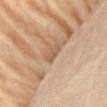Imaged during a routine full-body skin examination; the lesion was not biopsied and no histopathology is available. The lesion is on the abdomen. A 15 mm close-up tile from a total-body photography series done for melanoma screening. A female patient aged 78 to 82. The lesion's longest dimension is about 3 mm.